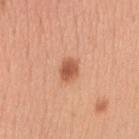Assessment:
The lesion was photographed on a routine skin check and not biopsied; there is no pathology result.
Acquisition and patient details:
The patient is a female aged 28 to 32. Automated tile analysis of the lesion measured lesion-presence confidence of about 100/100. Cropped from a whole-body photographic skin survey; the tile spans about 15 mm. From the right upper arm.